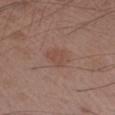Assessment:
This lesion was catalogued during total-body skin photography and was not selected for biopsy.
Background:
A close-up tile cropped from a whole-body skin photograph, about 15 mm across. An algorithmic analysis of the crop reported a footprint of about 5 mm², an outline eccentricity of about 0.6 (0 = round, 1 = elongated), and a symmetry-axis asymmetry near 0.3. It also reported about 6 CIELAB-L* units darker than the surrounding skin. The software also gave a border-irregularity rating of about 3/10 and a color-variation rating of about 2/10. The analysis additionally found a nevus-likeness score of about 10/100 and a lesion-detection confidence of about 100/100. Imaged with white-light lighting. Measured at roughly 3 mm in maximum diameter. On the left forearm. The patient is a male approximately 55 years of age.